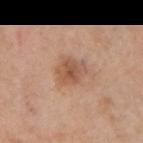| feature | finding |
|---|---|
| follow-up | catalogued during a skin exam; not biopsied |
| tile lighting | white-light illumination |
| acquisition | ~15 mm crop, total-body skin-cancer survey |
| patient | female, in their mid- to late 60s |
| location | the arm |